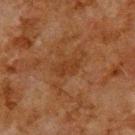Captured during whole-body skin photography for melanoma surveillance; the lesion was not biopsied.
A male subject, aged around 80.
Captured under cross-polarized illumination.
The lesion is located on the upper back.
The recorded lesion diameter is about 3 mm.
Cropped from a whole-body photographic skin survey; the tile spans about 15 mm.